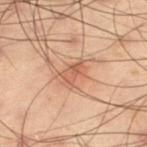| feature | finding |
|---|---|
| workup | imaged on a skin check; not biopsied |
| subject | male, in their mid- to late 50s |
| TBP lesion metrics | a border-irregularity index near 3/10, internal color variation of about 3 on a 0–10 scale, and a peripheral color-asymmetry measure near 1 |
| image source | total-body-photography crop, ~15 mm field of view |
| anatomic site | the left thigh |
| lighting | cross-polarized |